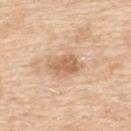A female subject, about 55 years old.
From the upper back.
The lesion's longest dimension is about 3.5 mm.
Cropped from a total-body skin-imaging series; the visible field is about 15 mm.
Automated image analysis of the tile measured a footprint of about 6.5 mm², an outline eccentricity of about 0.75 (0 = round, 1 = elongated), and a shape-asymmetry score of about 0.25 (0 = symmetric). The analysis additionally found an average lesion color of about L≈63 a*≈20 b*≈34 (CIELAB) and about 11 CIELAB-L* units darker than the surrounding skin. The analysis additionally found border irregularity of about 3 on a 0–10 scale and a within-lesion color-variation index near 2/10. The analysis additionally found a detector confidence of about 100 out of 100 that the crop contains a lesion.
This is a white-light tile.